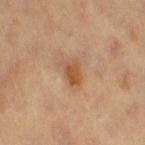Notes:
- biopsy status — imaged on a skin check; not biopsied
- location — the right thigh
- patient — female, about 70 years old
- size — ~3.5 mm (longest diameter)
- image source — ~15 mm crop, total-body skin-cancer survey